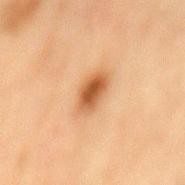Q: What is the lesion's diameter?
A: ~4 mm (longest diameter)
Q: Where on the body is the lesion?
A: the mid back
Q: Who is the patient?
A: female, roughly 80 years of age
Q: What is the imaging modality?
A: ~15 mm tile from a whole-body skin photo
Q: How was the tile lit?
A: cross-polarized